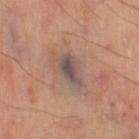No biopsy was performed on this lesion — it was imaged during a full skin examination and was not determined to be concerning. A 15 mm close-up tile from a total-body photography series done for melanoma screening. Automated tile analysis of the lesion measured a footprint of about 5.5 mm², a shape eccentricity near 0.85, and a symmetry-axis asymmetry near 0.3. Located on the left thigh. Imaged with cross-polarized lighting.